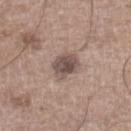Recorded during total-body skin imaging; not selected for excision or biopsy.
Imaged with white-light lighting.
Longest diameter approximately 4 mm.
An algorithmic analysis of the crop reported a classifier nevus-likeness of about 15/100 and a lesion-detection confidence of about 100/100.
Located on the left lower leg.
A close-up tile cropped from a whole-body skin photograph, about 15 mm across.
A male subject in their mid- to late 60s.